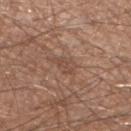Impression:
Captured during whole-body skin photography for melanoma surveillance; the lesion was not biopsied.
Background:
From the leg. A 15 mm close-up tile from a total-body photography series done for melanoma screening. The patient is a male roughly 65 years of age. This is a white-light tile. Longest diameter approximately 3 mm.